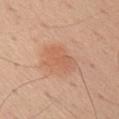workup: no biopsy performed (imaged during a skin exam); diameter: about 4.5 mm; subject: male, aged 53–57; illumination: white-light illumination; site: the right upper arm; acquisition: total-body-photography crop, ~15 mm field of view.